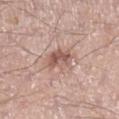{"site": "right lower leg", "patient": {"sex": "male", "age_approx": 40}, "lighting": "white-light", "lesion_size": {"long_diameter_mm_approx": 4.0}, "image": {"source": "total-body photography crop", "field_of_view_mm": 15}, "automated_metrics": {"cielab_L": 56, "cielab_a": 21, "cielab_b": 24, "vs_skin_darker_L": 11.0, "vs_skin_contrast_norm": 7.5, "nevus_likeness_0_100": 5}}